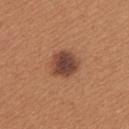notes: imaged on a skin check; not biopsied | patient: female, approximately 40 years of age | automated lesion analysis: a lesion area of about 9 mm², an eccentricity of roughly 0.5, and a shape-asymmetry score of about 0.2 (0 = symmetric); an average lesion color of about L≈44 a*≈23 b*≈28 (CIELAB) and a normalized border contrast of about 11; internal color variation of about 4.5 on a 0–10 scale and a peripheral color-asymmetry measure near 1; a classifier nevus-likeness of about 70/100 and lesion-presence confidence of about 100/100 | site: the left upper arm | illumination: white-light | image source: ~15 mm crop, total-body skin-cancer survey | lesion diameter: ~3.5 mm (longest diameter).